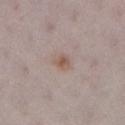This lesion was catalogued during total-body skin photography and was not selected for biopsy. Imaged with white-light lighting. The lesion's longest dimension is about 2.5 mm. The lesion is on the right lower leg. A female subject, about 30 years old. The lesion-visualizer software estimated an area of roughly 3.5 mm², a shape eccentricity near 0.75, and a shape-asymmetry score of about 0.25 (0 = symmetric). And it measured a lesion color around L≈55 a*≈16 b*≈24 in CIELAB, a lesion–skin lightness drop of about 8, and a normalized border contrast of about 7. It also reported an automated nevus-likeness rating near 75 out of 100 and a detector confidence of about 100 out of 100 that the crop contains a lesion. A 15 mm close-up extracted from a 3D total-body photography capture.